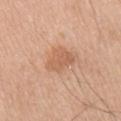No biopsy was performed on this lesion — it was imaged during a full skin examination and was not determined to be concerning. A male subject, aged approximately 70. Located on the right upper arm. About 3.5 mm across. A 15 mm crop from a total-body photograph taken for skin-cancer surveillance. This is a white-light tile.